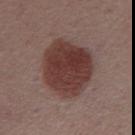biopsy status = imaged on a skin check; not biopsied
patient = male, about 55 years old
illumination = white-light illumination
lesion diameter = ~7 mm (longest diameter)
image source = total-body-photography crop, ~15 mm field of view
anatomic site = the front of the torso